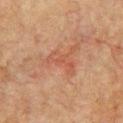• follow-up: imaged on a skin check; not biopsied
• acquisition: total-body-photography crop, ~15 mm field of view
• subject: male, roughly 75 years of age
• lesion size: ≈4 mm
• automated lesion analysis: a lesion area of about 6 mm² and an outline eccentricity of about 0.9 (0 = round, 1 = elongated); border irregularity of about 6 on a 0–10 scale and internal color variation of about 2.5 on a 0–10 scale
• site: the left upper arm